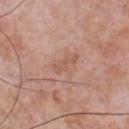workup=total-body-photography surveillance lesion; no biopsy
TBP lesion metrics=a color-variation rating of about 0/10 and peripheral color asymmetry of about 0; a classifier nevus-likeness of about 0/100 and lesion-presence confidence of about 100/100
location=the chest
imaging modality=~15 mm tile from a whole-body skin photo
tile lighting=white-light
lesion diameter=~3.5 mm (longest diameter)
patient=male, approximately 60 years of age